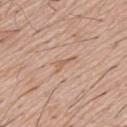biopsy_status: not biopsied; imaged during a skin examination
image:
  source: total-body photography crop
  field_of_view_mm: 15
automated_metrics:
  area_mm2_approx: 3.0
  shape_asymmetry: 0.65
  nevus_likeness_0_100: 0
lesion_size:
  long_diameter_mm_approx: 3.0
patient:
  sex: male
  age_approx: 55
lighting: white-light